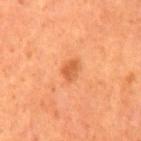follow-up: catalogued during a skin exam; not biopsied
image: ~15 mm crop, total-body skin-cancer survey
location: the mid back
patient: male, aged 63–67